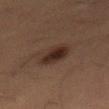Q: Was this lesion biopsied?
A: total-body-photography surveillance lesion; no biopsy
Q: Where on the body is the lesion?
A: the front of the torso
Q: Who is the patient?
A: male, aged approximately 55
Q: What did automated image analysis measure?
A: a lesion color around L≈24 a*≈14 b*≈19 in CIELAB, a lesion–skin lightness drop of about 8, and a normalized lesion–skin contrast near 9; border irregularity of about 2.5 on a 0–10 scale, a within-lesion color-variation index near 5/10, and a peripheral color-asymmetry measure near 1.5
Q: What kind of image is this?
A: 15 mm crop, total-body photography